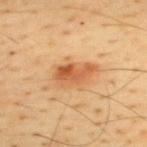Clinical impression:
No biopsy was performed on this lesion — it was imaged during a full skin examination and was not determined to be concerning.
Image and clinical context:
The lesion is located on the back. A region of skin cropped from a whole-body photographic capture, roughly 15 mm wide. A male patient, aged 43–47.